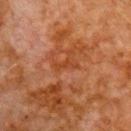Clinical impression: Captured during whole-body skin photography for melanoma surveillance; the lesion was not biopsied. Clinical summary: A roughly 15 mm field-of-view crop from a total-body skin photograph. The subject is a male about 80 years old. Located on the chest. The tile uses cross-polarized illumination. Longest diameter approximately 3 mm.